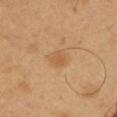acquisition — ~15 mm tile from a whole-body skin photo | patient — male, in their 50s | body site — the arm | size — ~2.5 mm (longest diameter).